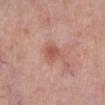From the right lower leg. Automated tile analysis of the lesion measured a footprint of about 5 mm², an outline eccentricity of about 0.6 (0 = round, 1 = elongated), and a symmetry-axis asymmetry near 0.15. It also reported a border-irregularity index near 1.5/10, a color-variation rating of about 2.5/10, and a peripheral color-asymmetry measure near 1. The analysis additionally found a detector confidence of about 100 out of 100 that the crop contains a lesion. This image is a 15 mm lesion crop taken from a total-body photograph. A female subject, aged around 55. The tile uses white-light illumination. Approximately 2.5 mm at its widest.